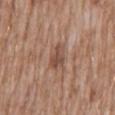No biopsy was performed on this lesion — it was imaged during a full skin examination and was not determined to be concerning. A 15 mm close-up tile from a total-body photography series done for melanoma screening. On the abdomen. A male patient, aged 58–62.